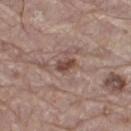Clinical impression: The lesion was photographed on a routine skin check and not biopsied; there is no pathology result. Image and clinical context: A male patient, about 65 years old. Cropped from a whole-body photographic skin survey; the tile spans about 15 mm. The lesion is located on the right thigh. Imaged with white-light lighting. Measured at roughly 2.5 mm in maximum diameter.